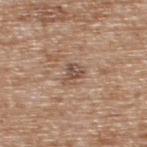* notes — imaged on a skin check; not biopsied
* image — total-body-photography crop, ~15 mm field of view
* tile lighting — white-light
* TBP lesion metrics — a lesion area of about 4 mm², a shape eccentricity near 0.65, and a shape-asymmetry score of about 0.4 (0 = symmetric); a mean CIELAB color near L≈51 a*≈17 b*≈26 and a lesion–skin lightness drop of about 10
* anatomic site — the upper back
* patient — male, in their mid- to late 60s
* diameter — ~2.5 mm (longest diameter)